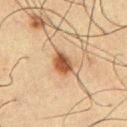notes = no biopsy performed (imaged during a skin exam)
image source = ~15 mm tile from a whole-body skin photo
tile lighting = cross-polarized illumination
patient = male, approximately 60 years of age
anatomic site = the front of the torso
lesion size = ~3.5 mm (longest diameter)
automated metrics = an area of roughly 5.5 mm² and a shape eccentricity near 0.7; an automated nevus-likeness rating near 95 out of 100 and a lesion-detection confidence of about 100/100